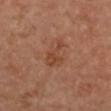Imaged during a routine full-body skin examination; the lesion was not biopsied and no histopathology is available. Longest diameter approximately 4 mm. A lesion tile, about 15 mm wide, cut from a 3D total-body photograph. An algorithmic analysis of the crop reported an eccentricity of roughly 0.8 and a shape-asymmetry score of about 0.55 (0 = symmetric). And it measured a border-irregularity index near 6/10 and internal color variation of about 2 on a 0–10 scale. A female patient, approximately 65 years of age. On the right upper arm.